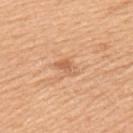biopsy status = imaged on a skin check; not biopsied
anatomic site = the left upper arm
size = ≈3 mm
subject = male, roughly 50 years of age
illumination = white-light
image-analysis metrics = an area of roughly 3.5 mm², an eccentricity of roughly 0.8, and two-axis asymmetry of about 0.4; a border-irregularity index near 3.5/10, a within-lesion color-variation index near 2/10, and peripheral color asymmetry of about 0.5
image source = total-body-photography crop, ~15 mm field of view